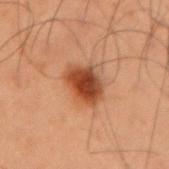No biopsy was performed on this lesion — it was imaged during a full skin examination and was not determined to be concerning.
The lesion-visualizer software estimated a mean CIELAB color near L≈38 a*≈23 b*≈31, roughly 13 lightness units darker than nearby skin, and a normalized lesion–skin contrast near 10.5.
Cropped from a whole-body photographic skin survey; the tile spans about 15 mm.
The lesion is on the arm.
The subject is a male in their mid- to late 50s.